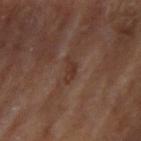The lesion was tiled from a total-body skin photograph and was not biopsied. This is a cross-polarized tile. A male subject, about 85 years old or older. The recorded lesion diameter is about 3 mm. The lesion is on the right upper arm. A lesion tile, about 15 mm wide, cut from a 3D total-body photograph.